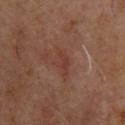Findings:
* workup — catalogued during a skin exam; not biopsied
* image source — ~15 mm crop, total-body skin-cancer survey
* subject — male, approximately 65 years of age
* anatomic site — the upper back